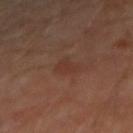Assessment:
Captured during whole-body skin photography for melanoma surveillance; the lesion was not biopsied.
Clinical summary:
The subject is a male aged 48–52. This image is a 15 mm lesion crop taken from a total-body photograph. Located on the arm.